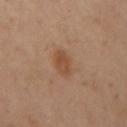The lesion was tiled from a total-body skin photograph and was not biopsied.
The tile uses cross-polarized illumination.
The lesion-visualizer software estimated a lesion area of about 6 mm², a shape eccentricity near 0.7, and a symmetry-axis asymmetry near 0.15.
The subject is a female aged 38–42.
Measured at roughly 3 mm in maximum diameter.
A close-up tile cropped from a whole-body skin photograph, about 15 mm across.
The lesion is located on the left arm.